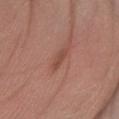{"biopsy_status": "not biopsied; imaged during a skin examination", "lesion_size": {"long_diameter_mm_approx": 2.5}, "image": {"source": "total-body photography crop", "field_of_view_mm": 15}, "patient": {"sex": "male", "age_approx": 70}, "site": "left forearm"}